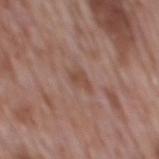Assessment: Captured during whole-body skin photography for melanoma surveillance; the lesion was not biopsied.